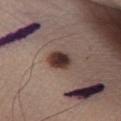Part of a total-body skin-imaging series; this lesion was reviewed on a skin check and was not flagged for biopsy. The tile uses white-light illumination. The lesion is on the leg. A male patient about 35 years old. The total-body-photography lesion software estimated a footprint of about 6.5 mm² and an eccentricity of roughly 0.55. The software also gave a nevus-likeness score of about 95/100. A region of skin cropped from a whole-body photographic capture, roughly 15 mm wide.